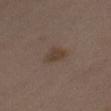<record>
  <biopsy_status>not biopsied; imaged during a skin examination</biopsy_status>
  <automated_metrics>
    <cielab_L>39</cielab_L>
    <cielab_a>13</cielab_a>
    <cielab_b>24</cielab_b>
    <vs_skin_darker_L>7.0</vs_skin_darker_L>
    <vs_skin_contrast_norm>7.0</vs_skin_contrast_norm>
  </automated_metrics>
  <patient>
    <sex>female</sex>
    <age_approx>35</age_approx>
  </patient>
  <site>abdomen</site>
  <lighting>white-light</lighting>
  <image>
    <source>total-body photography crop</source>
    <field_of_view_mm>15</field_of_view_mm>
  </image>
  <lesion_size>
    <long_diameter_mm_approx>3.0</long_diameter_mm_approx>
  </lesion_size>
</record>